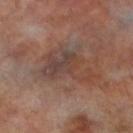Clinical impression: Part of a total-body skin-imaging series; this lesion was reviewed on a skin check and was not flagged for biopsy. Clinical summary: From the left lower leg. A 15 mm crop from a total-body photograph taken for skin-cancer surveillance. A male patient aged 68 to 72.